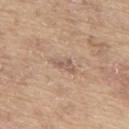follow-up: no biopsy performed (imaged during a skin exam)
illumination: white-light
image source: total-body-photography crop, ~15 mm field of view
image-analysis metrics: a mean CIELAB color near L≈57 a*≈17 b*≈26, a lesion–skin lightness drop of about 9, and a normalized border contrast of about 6
patient: male, about 65 years old
location: the upper back